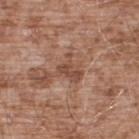biopsy_status: not biopsied; imaged during a skin examination
lighting: white-light
lesion_size:
  long_diameter_mm_approx: 3.5
image:
  source: total-body photography crop
  field_of_view_mm: 15
patient:
  sex: male
  age_approx: 55
site: upper back